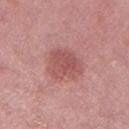The lesion was tiled from a total-body skin photograph and was not biopsied. The lesion is located on the left thigh. Imaged with white-light lighting. A female patient, aged 38 to 42. Automated tile analysis of the lesion measured an outline eccentricity of about 0.55 (0 = round, 1 = elongated) and a symmetry-axis asymmetry near 0.25. The software also gave an average lesion color of about L≈53 a*≈28 b*≈23 (CIELAB), roughly 9 lightness units darker than nearby skin, and a normalized border contrast of about 6. The recorded lesion diameter is about 4 mm. Cropped from a whole-body photographic skin survey; the tile spans about 15 mm.